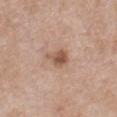notes — imaged on a skin check; not biopsied | image source — 15 mm crop, total-body photography | body site — the chest | patient — female, aged 38 to 42.